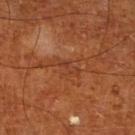Captured during whole-body skin photography for melanoma surveillance; the lesion was not biopsied.
Automated tile analysis of the lesion measured a lesion area of about 3 mm² and a symmetry-axis asymmetry near 0.35. The software also gave a peripheral color-asymmetry measure near 0. And it measured a detector confidence of about 85 out of 100 that the crop contains a lesion.
Longest diameter approximately 3.5 mm.
Located on the leg.
The tile uses cross-polarized illumination.
The subject is a male in their mid-60s.
A roughly 15 mm field-of-view crop from a total-body skin photograph.